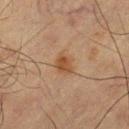Impression: Imaged during a routine full-body skin examination; the lesion was not biopsied and no histopathology is available. Image and clinical context: A region of skin cropped from a whole-body photographic capture, roughly 15 mm wide. The total-body-photography lesion software estimated a footprint of about 4.5 mm², an outline eccentricity of about 0.65 (0 = round, 1 = elongated), and a shape-asymmetry score of about 0.25 (0 = symmetric). The analysis additionally found a classifier nevus-likeness of about 80/100 and a lesion-detection confidence of about 100/100. A male patient, aged approximately 65. The lesion is on the right thigh.